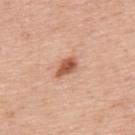Assessment: Captured during whole-body skin photography for melanoma surveillance; the lesion was not biopsied. Image and clinical context: Approximately 3 mm at its widest. Automated tile analysis of the lesion measured a lesion area of about 4.5 mm² and an eccentricity of roughly 0.8. The analysis additionally found a lesion color around L≈57 a*≈25 b*≈34 in CIELAB, a lesion–skin lightness drop of about 14, and a normalized border contrast of about 9.5. And it measured border irregularity of about 2.5 on a 0–10 scale, a within-lesion color-variation index near 3.5/10, and a peripheral color-asymmetry measure near 1. From the upper back. This is a white-light tile. A male patient aged approximately 75. A 15 mm close-up extracted from a 3D total-body photography capture.